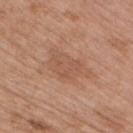Impression: Part of a total-body skin-imaging series; this lesion was reviewed on a skin check and was not flagged for biopsy. Context: A female subject about 40 years old. On the right upper arm. The lesion-visualizer software estimated a lesion area of about 12 mm². The software also gave a normalized border contrast of about 5. It also reported an automated nevus-likeness rating near 0 out of 100 and a lesion-detection confidence of about 100/100. Approximately 5.5 mm at its widest. Cropped from a total-body skin-imaging series; the visible field is about 15 mm.